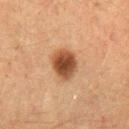The lesion was photographed on a routine skin check and not biopsied; there is no pathology result.
A lesion tile, about 15 mm wide, cut from a 3D total-body photograph.
The subject is a female approximately 55 years of age.
The lesion is located on the left forearm.